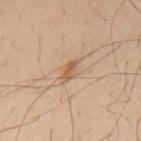Clinical impression: The lesion was photographed on a routine skin check and not biopsied; there is no pathology result. Image and clinical context: A male subject, about 50 years old. This image is a 15 mm lesion crop taken from a total-body photograph. The lesion is on the upper back. Approximately 3 mm at its widest. Captured under cross-polarized illumination.